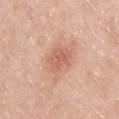notes = imaged on a skin check; not biopsied
imaging modality = ~15 mm crop, total-body skin-cancer survey
patient = male, about 45 years old
location = the chest